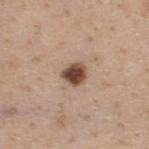The lesion was tiled from a total-body skin photograph and was not biopsied. Cropped from a total-body skin-imaging series; the visible field is about 15 mm. The subject is a male aged around 40. The lesion's longest dimension is about 2.5 mm. On the upper back. Captured under white-light illumination. Automated tile analysis of the lesion measured border irregularity of about 2 on a 0–10 scale, internal color variation of about 4.5 on a 0–10 scale, and a peripheral color-asymmetry measure near 1.5. And it measured a nevus-likeness score of about 100/100 and lesion-presence confidence of about 100/100.